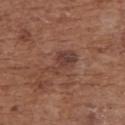– tile lighting · white-light illumination
– lesion diameter · about 4 mm
– anatomic site · the back
– image source · 15 mm crop, total-body photography
– patient · female, about 75 years old